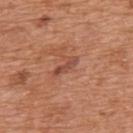Q: Is there a histopathology result?
A: total-body-photography surveillance lesion; no biopsy
Q: Who is the patient?
A: male, approximately 65 years of age
Q: What is the lesion's diameter?
A: about 3.5 mm
Q: Where on the body is the lesion?
A: the mid back
Q: Automated lesion metrics?
A: an average lesion color of about L≈48 a*≈25 b*≈30 (CIELAB) and a normalized border contrast of about 7; a border-irregularity rating of about 3.5/10 and a within-lesion color-variation index near 0/10
Q: What lighting was used for the tile?
A: white-light
Q: How was this image acquired?
A: ~15 mm tile from a whole-body skin photo